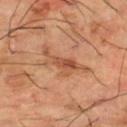workup — imaged on a skin check; not biopsied
image — ~15 mm tile from a whole-body skin photo
tile lighting — cross-polarized illumination
patient — male, aged approximately 65
location — the leg